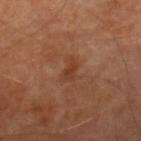follow-up: catalogued during a skin exam; not biopsied
TBP lesion metrics: an average lesion color of about L≈38 a*≈22 b*≈31 (CIELAB), a lesion–skin lightness drop of about 6, and a lesion-to-skin contrast of about 5.5 (normalized; higher = more distinct)
image: ~15 mm tile from a whole-body skin photo
patient: male, aged 68–72
diameter: ~2.5 mm (longest diameter)
site: the right lower leg